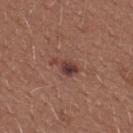Case summary:
* workup · total-body-photography surveillance lesion; no biopsy
* tile lighting · white-light
* anatomic site · the back
* subject · female, aged 23 to 27
* lesion size · about 3.5 mm
* imaging modality · 15 mm crop, total-body photography